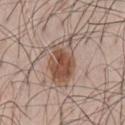The lesion was tiled from a total-body skin photograph and was not biopsied. A 15 mm close-up extracted from a 3D total-body photography capture. This is a white-light tile. Measured at roughly 6.5 mm in maximum diameter. On the chest. A male patient, in their 50s.